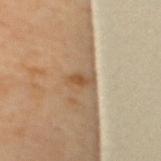| key | value |
|---|---|
| notes | no biopsy performed (imaged during a skin exam) |
| imaging modality | ~15 mm crop, total-body skin-cancer survey |
| patient | female, approximately 65 years of age |
| location | the back |